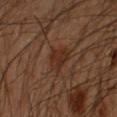follow-up = no biopsy performed (imaged during a skin exam) | subject = male, in their 50s | acquisition = ~15 mm tile from a whole-body skin photo | site = the arm | tile lighting = cross-polarized | lesion size = about 3 mm.